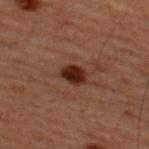| feature | finding |
|---|---|
| biopsy status | total-body-photography surveillance lesion; no biopsy |
| image | ~15 mm crop, total-body skin-cancer survey |
| tile lighting | cross-polarized |
| image-analysis metrics | a lesion area of about 4.5 mm², a shape eccentricity near 0.6, and two-axis asymmetry of about 0.2; a lesion-to-skin contrast of about 13.5 (normalized; higher = more distinct); internal color variation of about 2 on a 0–10 scale and radial color variation of about 0.5; an automated nevus-likeness rating near 95 out of 100 and a lesion-detection confidence of about 100/100 |
| site | the upper back |
| diameter | about 2.5 mm |
| patient | male, aged approximately 60 |